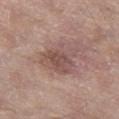<record>
<biopsy_status>not biopsied; imaged during a skin examination</biopsy_status>
<image>
  <source>total-body photography crop</source>
  <field_of_view_mm>15</field_of_view_mm>
</image>
<lighting>white-light</lighting>
<automated_metrics>
  <area_mm2_approx>12.0</area_mm2_approx>
  <eccentricity>0.35</eccentricity>
  <shape_asymmetry>0.3</shape_asymmetry>
  <lesion_detection_confidence_0_100>100</lesion_detection_confidence_0_100>
</automated_metrics>
<patient>
  <sex>male</sex>
  <age_approx>65</age_approx>
</patient>
<site>leg</site>
</record>